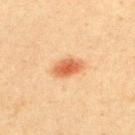Findings:
- anatomic site — the upper back
- patient — female, in their 40s
- tile lighting — cross-polarized
- imaging modality — total-body-photography crop, ~15 mm field of view
- diameter — ~3.5 mm (longest diameter)